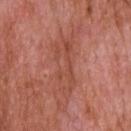Q: What did automated image analysis measure?
A: about 7 CIELAB-L* units darker than the surrounding skin and a normalized lesion–skin contrast near 5.5; a classifier nevus-likeness of about 0/100 and lesion-presence confidence of about 70/100
Q: What is the anatomic site?
A: the upper back
Q: How large is the lesion?
A: ~6.5 mm (longest diameter)
Q: What is the imaging modality?
A: 15 mm crop, total-body photography
Q: Patient demographics?
A: male, aged 63 to 67